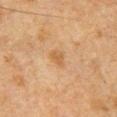Findings:
– notes · imaged on a skin check; not biopsied
– subject · male, aged 48 to 52
– acquisition · ~15 mm crop, total-body skin-cancer survey
– location · the chest